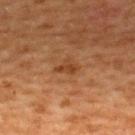Impression: Recorded during total-body skin imaging; not selected for excision or biopsy. Context: Automated tile analysis of the lesion measured an area of roughly 3.5 mm² and a shape-asymmetry score of about 0.4 (0 = symmetric). The software also gave roughly 7 lightness units darker than nearby skin and a lesion-to-skin contrast of about 6.5 (normalized; higher = more distinct). And it measured a classifier nevus-likeness of about 0/100. Imaged with cross-polarized lighting. A female patient about 40 years old. Cropped from a total-body skin-imaging series; the visible field is about 15 mm. From the upper back.